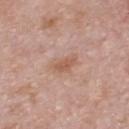notes: total-body-photography surveillance lesion; no biopsy
acquisition: ~15 mm crop, total-body skin-cancer survey
tile lighting: white-light illumination
location: the chest
lesion diameter: about 3 mm
patient: male, aged 53 to 57